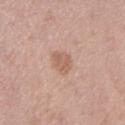The lesion was tiled from a total-body skin photograph and was not biopsied. A female patient, in their mid-60s. Located on the right lower leg. About 3 mm across. A region of skin cropped from a whole-body photographic capture, roughly 15 mm wide. Automated image analysis of the tile measured an area of roughly 5 mm² and an outline eccentricity of about 0.65 (0 = round, 1 = elongated). And it measured border irregularity of about 2.5 on a 0–10 scale, a within-lesion color-variation index near 2/10, and radial color variation of about 0.5. Captured under white-light illumination.